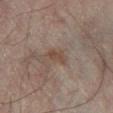The lesion was photographed on a routine skin check and not biopsied; there is no pathology result.
A male subject aged approximately 75.
The lesion is on the right lower leg.
A 15 mm close-up tile from a total-body photography series done for melanoma screening.